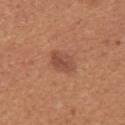* follow-up · no biopsy performed (imaged during a skin exam)
* acquisition · ~15 mm tile from a whole-body skin photo
* subject · female, about 40 years old
* size · ~3.5 mm (longest diameter)
* body site · the upper back
* tile lighting · white-light